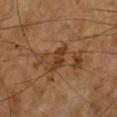This lesion was catalogued during total-body skin photography and was not selected for biopsy.
The lesion-visualizer software estimated an area of roughly 4 mm² and a symmetry-axis asymmetry near 0.3. And it measured a classifier nevus-likeness of about 0/100 and lesion-presence confidence of about 65/100.
Cropped from a whole-body photographic skin survey; the tile spans about 15 mm.
The lesion is located on the left lower leg.
Captured under cross-polarized illumination.
A male subject aged approximately 65.